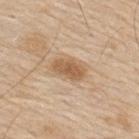{
  "lighting": "white-light",
  "image": {
    "source": "total-body photography crop",
    "field_of_view_mm": 15
  },
  "patient": {
    "sex": "male",
    "age_approx": 80
  },
  "site": "back",
  "automated_metrics": {
    "area_mm2_approx": 7.5,
    "eccentricity": 0.85,
    "shape_asymmetry": 0.15,
    "color_variation_0_10": 2.5,
    "peripheral_color_asymmetry": 1.0,
    "lesion_detection_confidence_0_100": 100
  },
  "lesion_size": {
    "long_diameter_mm_approx": 4.0
  }
}